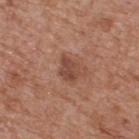The lesion was tiled from a total-body skin photograph and was not biopsied.
Imaged with white-light lighting.
Cropped from a total-body skin-imaging series; the visible field is about 15 mm.
The patient is a male about 65 years old.
The lesion-visualizer software estimated a lesion color around L≈46 a*≈23 b*≈27 in CIELAB and a normalized border contrast of about 7. The analysis additionally found a lesion-detection confidence of about 100/100.
Located on the upper back.
The recorded lesion diameter is about 2.5 mm.